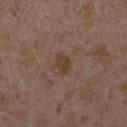Clinical summary:
Imaged with white-light lighting. Located on the right forearm. The subject is a female in their mid- to late 30s. A 15 mm close-up extracted from a 3D total-body photography capture.